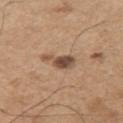<lesion>
<biopsy_status>not biopsied; imaged during a skin examination</biopsy_status>
<image>
  <source>total-body photography crop</source>
  <field_of_view_mm>15</field_of_view_mm>
</image>
<lighting>white-light</lighting>
<site>left upper arm</site>
<automated_metrics>
  <cielab_L>49</cielab_L>
  <cielab_a>19</cielab_a>
  <cielab_b>29</cielab_b>
  <vs_skin_darker_L>14.0</vs_skin_darker_L>
  <vs_skin_contrast_norm>10.0</vs_skin_contrast_norm>
</automated_metrics>
<patient>
  <sex>male</sex>
  <age_approx>65</age_approx>
</patient>
</lesion>